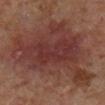This lesion was catalogued during total-body skin photography and was not selected for biopsy.
A male subject, in their 60s.
A close-up tile cropped from a whole-body skin photograph, about 15 mm across.
The recorded lesion diameter is about 13.5 mm.
From the right lower leg.
Captured under cross-polarized illumination.